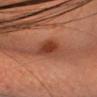automated metrics = a lesion area of about 6 mm² and an eccentricity of roughly 0.95; a border-irregularity index near 3/10, internal color variation of about 3.5 on a 0–10 scale, and radial color variation of about 1.5 | anatomic site = the head or neck | subject = female, aged around 35 | lesion diameter = ~4.5 mm (longest diameter) | image source = 15 mm crop, total-body photography.